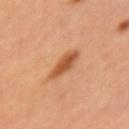{"biopsy_status": "not biopsied; imaged during a skin examination", "lesion_size": {"long_diameter_mm_approx": 3.5}, "lighting": "cross-polarized", "image": {"source": "total-body photography crop", "field_of_view_mm": 15}, "site": "left upper arm", "patient": {"sex": "female", "age_approx": 35}}